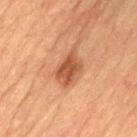Assessment:
Part of a total-body skin-imaging series; this lesion was reviewed on a skin check and was not flagged for biopsy.
Background:
Cropped from a total-body skin-imaging series; the visible field is about 15 mm. The lesion-visualizer software estimated an automated nevus-likeness rating near 75 out of 100 and lesion-presence confidence of about 100/100. A male patient, aged around 85 or older. Measured at roughly 3.5 mm in maximum diameter. Located on the back. Captured under cross-polarized illumination.